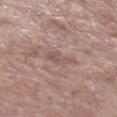{"biopsy_status": "not biopsied; imaged during a skin examination", "patient": {"sex": "female", "age_approx": "85+"}, "image": {"source": "total-body photography crop", "field_of_view_mm": 15}, "site": "right lower leg"}